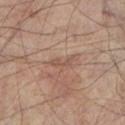<tbp_lesion>
  <biopsy_status>not biopsied; imaged during a skin examination</biopsy_status>
  <image>
    <source>total-body photography crop</source>
    <field_of_view_mm>15</field_of_view_mm>
  </image>
  <lesion_size>
    <long_diameter_mm_approx>3.0</long_diameter_mm_approx>
  </lesion_size>
  <lighting>cross-polarized</lighting>
  <site>left lower leg</site>
  <automated_metrics>
    <area_mm2_approx>2.5</area_mm2_approx>
    <eccentricity>0.95</eccentricity>
    <shape_asymmetry>0.55</shape_asymmetry>
    <cielab_L>49</cielab_L>
    <cielab_a>18</cielab_a>
    <cielab_b>25</cielab_b>
    <border_irregularity_0_10>6.0</border_irregularity_0_10>
    <color_variation_0_10>0.0</color_variation_0_10>
    <lesion_detection_confidence_0_100>65</lesion_detection_confidence_0_100>
  </automated_metrics>
  <patient>
    <sex>male</sex>
    <age_approx>65</age_approx>
  </patient>
</tbp_lesion>